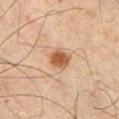Background: A male subject aged approximately 65. On the front of the torso. A region of skin cropped from a whole-body photographic capture, roughly 15 mm wide.